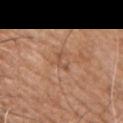workup: no biopsy performed (imaged during a skin exam) | diameter: ≈2.5 mm | TBP lesion metrics: an area of roughly 2 mm², an eccentricity of roughly 0.9, and a shape-asymmetry score of about 0.7 (0 = symmetric) | body site: the chest | patient: male, aged around 55 | image source: ~15 mm crop, total-body skin-cancer survey | illumination: white-light.